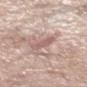Captured during whole-body skin photography for melanoma surveillance; the lesion was not biopsied. Approximately 3 mm at its widest. A 15 mm crop from a total-body photograph taken for skin-cancer surveillance. The patient is a female aged 83 or older. The lesion is located on the leg. The lesion-visualizer software estimated an area of roughly 4 mm², a shape eccentricity near 0.85, and a shape-asymmetry score of about 0.3 (0 = symmetric). The software also gave about 9 CIELAB-L* units darker than the surrounding skin and a normalized border contrast of about 5.5. The analysis additionally found a classifier nevus-likeness of about 0/100 and a lesion-detection confidence of about 80/100. This is a white-light tile.